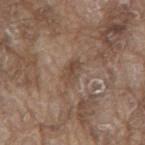Notes:
- size — ~3 mm (longest diameter)
- lighting — white-light illumination
- automated lesion analysis — a shape eccentricity near 0.8 and a shape-asymmetry score of about 0.25 (0 = symmetric); a border-irregularity rating of about 3/10 and radial color variation of about 1; a classifier nevus-likeness of about 0/100 and a detector confidence of about 95 out of 100 that the crop contains a lesion
- imaging modality — ~15 mm crop, total-body skin-cancer survey
- body site — the mid back
- subject — male, aged 78 to 82